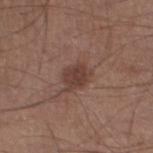workup=total-body-photography surveillance lesion; no biopsy
patient=male, approximately 45 years of age
lighting=white-light
lesion diameter=~3.5 mm (longest diameter)
acquisition=15 mm crop, total-body photography
image-analysis metrics=a lesion area of about 7 mm², a shape eccentricity near 0.65, and two-axis asymmetry of about 0.25; roughly 9 lightness units darker than nearby skin and a normalized lesion–skin contrast near 7.5; a border-irregularity index near 2.5/10, internal color variation of about 2 on a 0–10 scale, and radial color variation of about 0.5
location=the left lower leg